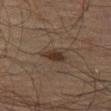– notes: catalogued during a skin exam; not biopsied
– lighting: cross-polarized illumination
– lesion size: ~3.5 mm (longest diameter)
– patient: male, roughly 70 years of age
– anatomic site: the left thigh
– automated metrics: a footprint of about 5.5 mm², a shape eccentricity near 0.8, and a shape-asymmetry score of about 0.25 (0 = symmetric); a lesion color around L≈27 a*≈12 b*≈20 in CIELAB, a lesion–skin lightness drop of about 7, and a normalized lesion–skin contrast near 8; an automated nevus-likeness rating near 85 out of 100 and lesion-presence confidence of about 100/100
– imaging modality: 15 mm crop, total-body photography